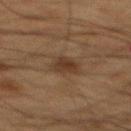Q: Was a biopsy performed?
A: catalogued during a skin exam; not biopsied
Q: What lighting was used for the tile?
A: cross-polarized illumination
Q: How large is the lesion?
A: ~3.5 mm (longest diameter)
Q: How was this image acquired?
A: ~15 mm tile from a whole-body skin photo
Q: Where on the body is the lesion?
A: the mid back
Q: Patient demographics?
A: male, aged 63–67